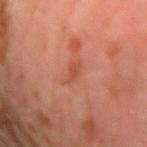workup = no biopsy performed (imaged during a skin exam)
illumination = cross-polarized
patient = male, aged approximately 60
location = the arm
image source = ~15 mm tile from a whole-body skin photo